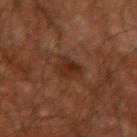The lesion was photographed on a routine skin check and not biopsied; there is no pathology result. This image is a 15 mm lesion crop taken from a total-body photograph. The patient is a male aged 58–62. Imaged with cross-polarized lighting. About 3 mm across. The lesion is located on the arm.